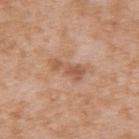Q: Is there a histopathology result?
A: no biopsy performed (imaged during a skin exam)
Q: Lesion location?
A: the back
Q: Lesion size?
A: ≈4 mm
Q: What is the imaging modality?
A: total-body-photography crop, ~15 mm field of view
Q: What are the patient's age and sex?
A: female, aged around 40
Q: Automated lesion metrics?
A: a border-irregularity rating of about 6/10 and a color-variation rating of about 1.5/10; a nevus-likeness score of about 0/100 and a lesion-detection confidence of about 100/100
Q: What lighting was used for the tile?
A: white-light